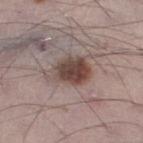The lesion was photographed on a routine skin check and not biopsied; there is no pathology result.
About 5 mm across.
The tile uses white-light illumination.
An algorithmic analysis of the crop reported a footprint of about 12 mm² and a symmetry-axis asymmetry near 0.2.
A male subject, aged 68 to 72.
This image is a 15 mm lesion crop taken from a total-body photograph.
Located on the right thigh.